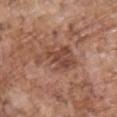  patient:
    sex: male
    age_approx: 70
  lesion_size:
    long_diameter_mm_approx: 6.0
  site: chest
  image:
    source: total-body photography crop
    field_of_view_mm: 15